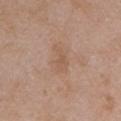This lesion was catalogued during total-body skin photography and was not selected for biopsy. Imaged with white-light lighting. Automated tile analysis of the lesion measured an area of roughly 4.5 mm², a shape eccentricity near 0.9, and a symmetry-axis asymmetry near 0.4. And it measured a mean CIELAB color near L≈56 a*≈18 b*≈30 and roughly 6 lightness units darker than nearby skin. And it measured a nevus-likeness score of about 0/100 and lesion-presence confidence of about 100/100. On the left upper arm. Cropped from a total-body skin-imaging series; the visible field is about 15 mm. About 3.5 mm across. The patient is a female in their mid- to late 50s.